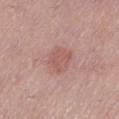Automated tile analysis of the lesion measured an outline eccentricity of about 0.7 (0 = round, 1 = elongated) and two-axis asymmetry of about 0.5. The analysis additionally found a lesion–skin lightness drop of about 7. The analysis additionally found a border-irregularity index near 5/10, a within-lesion color-variation index near 2/10, and radial color variation of about 0.5. It also reported a classifier nevus-likeness of about 0/100 and a detector confidence of about 100 out of 100 that the crop contains a lesion. Captured under white-light illumination. The patient is a female in their mid- to late 60s. Measured at roughly 3 mm in maximum diameter. On the right lower leg. Cropped from a total-body skin-imaging series; the visible field is about 15 mm.